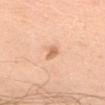  automated_metrics:
    color_variation_0_10: 1.0
    peripheral_color_asymmetry: 0.5
  lighting: white-light
  image:
    source: total-body photography crop
    field_of_view_mm: 15
  lesion_size:
    long_diameter_mm_approx: 2.5
  patient:
    sex: male
    age_approx: 60
  site: front of the torso